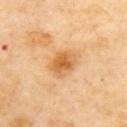• automated metrics: a lesion color around L≈64 a*≈24 b*≈46 in CIELAB and a normalized border contrast of about 8; a within-lesion color-variation index near 4.5/10 and peripheral color asymmetry of about 1; a classifier nevus-likeness of about 70/100
• size: ~3.5 mm (longest diameter)
• image source: ~15 mm crop, total-body skin-cancer survey
• subject: female, roughly 60 years of age
• tile lighting: cross-polarized
• anatomic site: the upper back